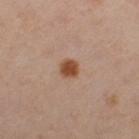The lesion was photographed on a routine skin check and not biopsied; there is no pathology result. The lesion's longest dimension is about 2 mm. A lesion tile, about 15 mm wide, cut from a 3D total-body photograph. Captured under cross-polarized illumination. The lesion is on the leg. An algorithmic analysis of the crop reported an average lesion color of about L≈48 a*≈22 b*≈32 (CIELAB) and roughly 12 lightness units darker than nearby skin. And it measured a border-irregularity rating of about 1.5/10, a color-variation rating of about 2.5/10, and a peripheral color-asymmetry measure near 0.5. And it measured a nevus-likeness score of about 100/100. A female subject, aged approximately 40.